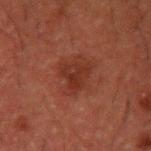The lesion is located on the left upper arm. Automated tile analysis of the lesion measured a shape-asymmetry score of about 0.45 (0 = symmetric). The software also gave a mean CIELAB color near L≈25 a*≈22 b*≈24, about 6 CIELAB-L* units darker than the surrounding skin, and a normalized lesion–skin contrast near 6.5. The analysis additionally found border irregularity of about 4 on a 0–10 scale and radial color variation of about 1. The software also gave an automated nevus-likeness rating near 0 out of 100 and a detector confidence of about 100 out of 100 that the crop contains a lesion. This is a cross-polarized tile. The patient is a male aged 48–52. Measured at roughly 3.5 mm in maximum diameter. A 15 mm close-up tile from a total-body photography series done for melanoma screening.